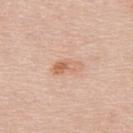workup — imaged on a skin check; not biopsied | subject — female, aged 43–47 | lighting — white-light illumination | size — about 3.5 mm | automated metrics — an average lesion color of about L≈65 a*≈22 b*≈33 (CIELAB) and a lesion–skin lightness drop of about 9; a border-irregularity rating of about 3.5/10, a color-variation rating of about 8/10, and radial color variation of about 3 | body site — the upper back | acquisition — ~15 mm crop, total-body skin-cancer survey.